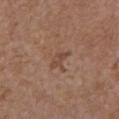| feature | finding |
|---|---|
| follow-up | no biopsy performed (imaged during a skin exam) |
| location | the front of the torso |
| diameter | about 3 mm |
| acquisition | total-body-photography crop, ~15 mm field of view |
| patient | female, aged 63–67 |
| tile lighting | white-light |
| automated metrics | an average lesion color of about L≈46 a*≈19 b*≈28 (CIELAB), roughly 7 lightness units darker than nearby skin, and a lesion-to-skin contrast of about 6 (normalized; higher = more distinct); a lesion-detection confidence of about 100/100 |